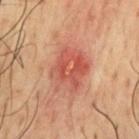Image and clinical context: Cropped from a whole-body photographic skin survey; the tile spans about 15 mm. The patient is a male aged around 50. Captured under cross-polarized illumination. From the chest.